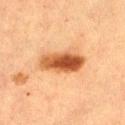Imaged with cross-polarized lighting.
Automated tile analysis of the lesion measured a border-irregularity index near 2.5/10 and a color-variation rating of about 8/10.
The lesion is on the right thigh.
Approximately 5.5 mm at its widest.
A 15 mm close-up extracted from a 3D total-body photography capture.
The patient is a female aged 53 to 57.